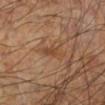notes: imaged on a skin check; not biopsied | body site: the left lower leg | tile lighting: cross-polarized illumination | size: about 3.5 mm | patient: male, approximately 60 years of age | image: ~15 mm crop, total-body skin-cancer survey | TBP lesion metrics: an area of roughly 4.5 mm² and an eccentricity of roughly 0.9; a lesion color around L≈44 a*≈19 b*≈31 in CIELAB, a lesion–skin lightness drop of about 7, and a normalized lesion–skin contrast near 5.5; a within-lesion color-variation index near 1.5/10; lesion-presence confidence of about 95/100.